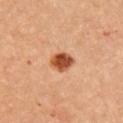Clinical impression:
The lesion was tiled from a total-body skin photograph and was not biopsied.
Clinical summary:
On the arm. Automated image analysis of the tile measured a shape eccentricity near 0.6 and a symmetry-axis asymmetry near 0.2. The software also gave a border-irregularity rating of about 1.5/10 and a color-variation rating of about 4.5/10. A lesion tile, about 15 mm wide, cut from a 3D total-body photograph. The patient is a female in their mid- to late 40s. The tile uses cross-polarized illumination.